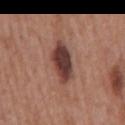workup = no biopsy performed (imaged during a skin exam); image source = ~15 mm crop, total-body skin-cancer survey; anatomic site = the mid back; subject = male, aged approximately 70.